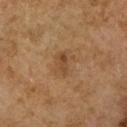{
  "lighting": "cross-polarized",
  "automated_metrics": {
    "cielab_L": 40,
    "cielab_a": 17,
    "cielab_b": 30,
    "vs_skin_darker_L": 7.0,
    "vs_skin_contrast_norm": 6.0,
    "nevus_likeness_0_100": 5,
    "lesion_detection_confidence_0_100": 100
  },
  "lesion_size": {
    "long_diameter_mm_approx": 3.0
  },
  "image": {
    "source": "total-body photography crop",
    "field_of_view_mm": 15
  },
  "patient": {
    "sex": "female",
    "age_approx": 60
  },
  "site": "arm"
}